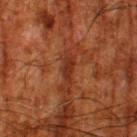<case>
<biopsy_status>not biopsied; imaged during a skin examination</biopsy_status>
<patient>
  <sex>male</sex>
  <age_approx>80</age_approx>
</patient>
<automated_metrics>
  <area_mm2_approx>3.5</area_mm2_approx>
  <eccentricity>0.9</eccentricity>
  <shape_asymmetry>0.35</shape_asymmetry>
  <nevus_likeness_0_100>0</nevus_likeness_0_100>
  <lesion_detection_confidence_0_100>90</lesion_detection_confidence_0_100>
</automated_metrics>
<lesion_size>
  <long_diameter_mm_approx>3.0</long_diameter_mm_approx>
</lesion_size>
<site>leg</site>
<lighting>cross-polarized</lighting>
<image>
  <source>total-body photography crop</source>
  <field_of_view_mm>15</field_of_view_mm>
</image>
</case>